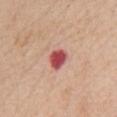follow-up — total-body-photography surveillance lesion; no biopsy
location — the front of the torso
image source — ~15 mm crop, total-body skin-cancer survey
lesion size — ~2.5 mm (longest diameter)
subject — female, aged around 65
tile lighting — white-light